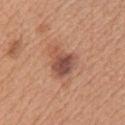Longest diameter approximately 4 mm.
Captured under white-light illumination.
Automated tile analysis of the lesion measured a lesion color around L≈51 a*≈23 b*≈28 in CIELAB and a normalized border contrast of about 8.5. The software also gave internal color variation of about 5.5 on a 0–10 scale and peripheral color asymmetry of about 1.5. The software also gave an automated nevus-likeness rating near 35 out of 100 and a detector confidence of about 100 out of 100 that the crop contains a lesion.
The lesion is on the chest.
Cropped from a whole-body photographic skin survey; the tile spans about 15 mm.
A female subject aged 43 to 47.